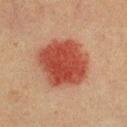The lesion was tiled from a total-body skin photograph and was not biopsied. A roughly 15 mm field-of-view crop from a total-body skin photograph. The subject is a male aged around 40. The lesion is on the chest.